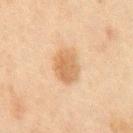Captured during whole-body skin photography for melanoma surveillance; the lesion was not biopsied.
A 15 mm crop from a total-body photograph taken for skin-cancer surveillance.
The lesion's longest dimension is about 3.5 mm.
This is a cross-polarized tile.
The subject is a male approximately 45 years of age.
The lesion is on the abdomen.